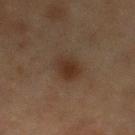This lesion was catalogued during total-body skin photography and was not selected for biopsy. The subject is a female aged around 55. Cropped from a total-body skin-imaging series; the visible field is about 15 mm. Automated image analysis of the tile measured a shape eccentricity near 0.5 and two-axis asymmetry of about 0.15. Approximately 3 mm at its widest. The lesion is located on the left lower leg. The tile uses cross-polarized illumination.